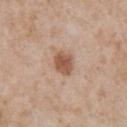Q: Was this lesion biopsied?
A: imaged on a skin check; not biopsied
Q: What are the patient's age and sex?
A: male, in their mid- to late 60s
Q: What is the imaging modality?
A: total-body-photography crop, ~15 mm field of view
Q: Lesion location?
A: the chest
Q: What is the lesion's diameter?
A: ~3 mm (longest diameter)
Q: Illumination type?
A: white-light